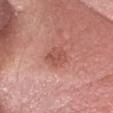Case summary:
- follow-up: catalogued during a skin exam; not biopsied
- acquisition: ~15 mm tile from a whole-body skin photo
- subject: male, aged 63 to 67
- body site: the head or neck
- illumination: white-light illumination